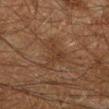biopsy_status: not biopsied; imaged during a skin examination
lighting: cross-polarized
image:
  source: total-body photography crop
  field_of_view_mm: 15
patient:
  sex: male
  age_approx: 60
site: left lower leg